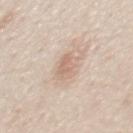The lesion was tiled from a total-body skin photograph and was not biopsied. Imaged with white-light lighting. A roughly 15 mm field-of-view crop from a total-body skin photograph. The total-body-photography lesion software estimated a lesion area of about 5 mm², a shape eccentricity near 0.85, and a shape-asymmetry score of about 0.25 (0 = symmetric). A male subject, about 35 years old. On the chest. Measured at roughly 3.5 mm in maximum diameter.